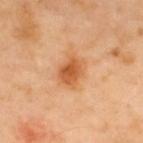Q: Is there a histopathology result?
A: catalogued during a skin exam; not biopsied
Q: What are the patient's age and sex?
A: male, aged around 40
Q: How large is the lesion?
A: about 3.5 mm
Q: What kind of image is this?
A: 15 mm crop, total-body photography
Q: What is the anatomic site?
A: the upper back